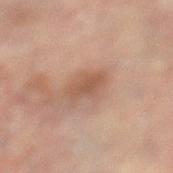The lesion was tiled from a total-body skin photograph and was not biopsied.
A roughly 15 mm field-of-view crop from a total-body skin photograph.
Captured under cross-polarized illumination.
On the right leg.
A female patient approximately 80 years of age.
Measured at roughly 3.5 mm in maximum diameter.